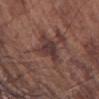| field | value |
|---|---|
| notes | no biopsy performed (imaged during a skin exam) |
| body site | the arm |
| lesion size | ≈5 mm |
| imaging modality | ~15 mm tile from a whole-body skin photo |
| patient | male, approximately 70 years of age |
| illumination | white-light |